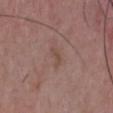workup = total-body-photography surveillance lesion; no biopsy
anatomic site = the chest
acquisition = ~15 mm crop, total-body skin-cancer survey
subject = male, aged 48 to 52